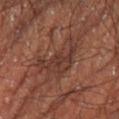This lesion was catalogued during total-body skin photography and was not selected for biopsy. This image is a 15 mm lesion crop taken from a total-body photograph. Measured at roughly 6.5 mm in maximum diameter. The subject is a male aged approximately 60. On the right thigh. An algorithmic analysis of the crop reported an area of roughly 18 mm² and a symmetry-axis asymmetry near 0.5. It also reported an average lesion color of about L≈33 a*≈19 b*≈23 (CIELAB), a lesion–skin lightness drop of about 6, and a normalized lesion–skin contrast near 6.